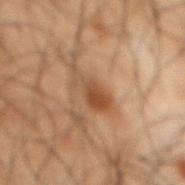A 15 mm close-up tile from a total-body photography series done for melanoma screening.
Located on the back.
The subject is a male in their 50s.
This is a cross-polarized tile.
About 4.5 mm across.